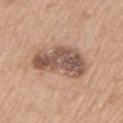This lesion was catalogued during total-body skin photography and was not selected for biopsy. On the left upper arm. Imaged with white-light lighting. Cropped from a total-body skin-imaging series; the visible field is about 15 mm. A male patient, about 70 years old. Longest diameter approximately 6.5 mm.